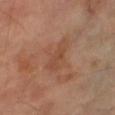Imaged during a routine full-body skin examination; the lesion was not biopsied and no histopathology is available. A male subject aged approximately 70. The lesion is on the right thigh. Cropped from a total-body skin-imaging series; the visible field is about 15 mm. The lesion's longest dimension is about 4.5 mm. This is a cross-polarized tile.